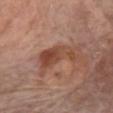The lesion was tiled from a total-body skin photograph and was not biopsied.